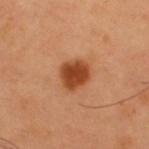Notes:
– biopsy status — no biopsy performed (imaged during a skin exam)
– lighting — cross-polarized illumination
– TBP lesion metrics — a lesion area of about 8 mm², an eccentricity of roughly 0.4, and two-axis asymmetry of about 0.2; a mean CIELAB color near L≈43 a*≈27 b*≈37, about 14 CIELAB-L* units darker than the surrounding skin, and a normalized lesion–skin contrast near 11; a nevus-likeness score of about 100/100 and a lesion-detection confidence of about 100/100
– patient — male, in their mid-50s
– imaging modality — ~15 mm tile from a whole-body skin photo
– lesion diameter — ~3.5 mm (longest diameter)
– location — the upper back